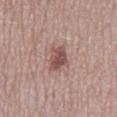biopsy status = no biopsy performed (imaged during a skin exam)
patient = male, aged approximately 75
size = ≈3.5 mm
automated metrics = an area of roughly 6 mm², a shape eccentricity near 0.8, and a symmetry-axis asymmetry near 0.25; roughly 12 lightness units darker than nearby skin and a normalized lesion–skin contrast near 8.5; internal color variation of about 3 on a 0–10 scale
site = the mid back
imaging modality = ~15 mm tile from a whole-body skin photo